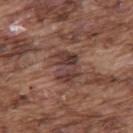follow-up: imaged on a skin check; not biopsied
subject: male, aged approximately 75
location: the upper back
lesion size: about 4 mm
acquisition: ~15 mm tile from a whole-body skin photo
lighting: white-light
image-analysis metrics: an area of roughly 11 mm², an eccentricity of roughly 0.6, and a shape-asymmetry score of about 0.2 (0 = symmetric); a lesion color around L≈39 a*≈19 b*≈22 in CIELAB and a normalized lesion–skin contrast near 8; a detector confidence of about 95 out of 100 that the crop contains a lesion